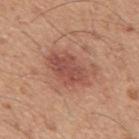A lesion tile, about 15 mm wide, cut from a 3D total-body photograph.
The lesion-visualizer software estimated a lesion color around L≈51 a*≈25 b*≈28 in CIELAB and a normalized border contrast of about 7. The analysis additionally found border irregularity of about 3.5 on a 0–10 scale, a color-variation rating of about 3.5/10, and peripheral color asymmetry of about 1.5. The analysis additionally found lesion-presence confidence of about 100/100.
Longest diameter approximately 5.5 mm.
The patient is a male aged approximately 45.
Imaged with white-light lighting.
From the back.